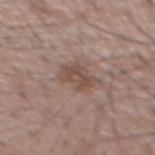biopsy status: total-body-photography surveillance lesion; no biopsy
illumination: white-light illumination
imaging modality: ~15 mm crop, total-body skin-cancer survey
size: ≈4 mm
location: the chest
TBP lesion metrics: a lesion area of about 7 mm², a shape eccentricity near 0.65, and a symmetry-axis asymmetry near 0.3; a border-irregularity rating of about 3.5/10, internal color variation of about 2.5 on a 0–10 scale, and peripheral color asymmetry of about 1
patient: male, roughly 65 years of age